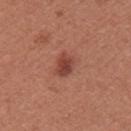| field | value |
|---|---|
| biopsy status | total-body-photography surveillance lesion; no biopsy |
| automated lesion analysis | an average lesion color of about L≈43 a*≈27 b*≈28 (CIELAB), about 11 CIELAB-L* units darker than the surrounding skin, and a lesion-to-skin contrast of about 8 (normalized; higher = more distinct); a nevus-likeness score of about 85/100 and a lesion-detection confidence of about 100/100 |
| image | ~15 mm crop, total-body skin-cancer survey |
| lesion size | ≈3 mm |
| location | the left upper arm |
| subject | female, approximately 25 years of age |
| lighting | white-light illumination |